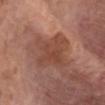Context:
The patient is a male roughly 80 years of age. The total-body-photography lesion software estimated a symmetry-axis asymmetry near 0.25. The analysis additionally found an average lesion color of about L≈46 a*≈24 b*≈28 (CIELAB). Approximately 5 mm at its widest. A 15 mm close-up extracted from a 3D total-body photography capture. This is a white-light tile. The lesion is located on the front of the torso.